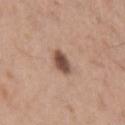• biopsy status · total-body-photography surveillance lesion; no biopsy
• automated lesion analysis · an eccentricity of roughly 0.5 and a shape-asymmetry score of about 0.25 (0 = symmetric); a mean CIELAB color near L≈50 a*≈19 b*≈26, roughly 15 lightness units darker than nearby skin, and a normalized border contrast of about 10.5; a border-irregularity index near 2/10 and a peripheral color-asymmetry measure near 1; an automated nevus-likeness rating near 90 out of 100 and a detector confidence of about 100 out of 100 that the crop contains a lesion
• tile lighting · white-light illumination
• lesion diameter · ≈2.5 mm
• subject · female, roughly 40 years of age
• imaging modality · ~15 mm crop, total-body skin-cancer survey
• body site · the left upper arm